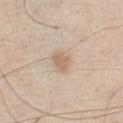A male subject in their mid-40s. A region of skin cropped from a whole-body photographic capture, roughly 15 mm wide. The lesion is located on the chest. Automated tile analysis of the lesion measured a lesion area of about 4.5 mm² and an eccentricity of roughly 0.65. The software also gave a normalized border contrast of about 6.5. And it measured an automated nevus-likeness rating near 75 out of 100 and a detector confidence of about 100 out of 100 that the crop contains a lesion. Captured under white-light illumination. Approximately 3 mm at its widest.